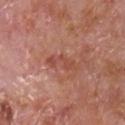Q: Is there a histopathology result?
A: total-body-photography surveillance lesion; no biopsy